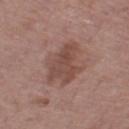Impression: No biopsy was performed on this lesion — it was imaged during a full skin examination and was not determined to be concerning. Background: A 15 mm close-up tile from a total-body photography series done for melanoma screening. A male subject aged around 55. Located on the leg.